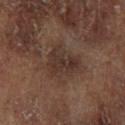Assessment:
The lesion was photographed on a routine skin check and not biopsied; there is no pathology result.
Clinical summary:
Cropped from a total-body skin-imaging series; the visible field is about 15 mm. A male patient, aged approximately 65. On the left lower leg. Captured under cross-polarized illumination. The lesion's longest dimension is about 4 mm.